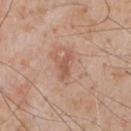notes — total-body-photography surveillance lesion; no biopsy | lighting — white-light | imaging modality — ~15 mm crop, total-body skin-cancer survey | subject — male, aged 58–62 | site — the chest | lesion diameter — about 3.5 mm.